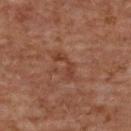Q: Is there a histopathology result?
A: total-body-photography surveillance lesion; no biopsy
Q: Lesion location?
A: the upper back
Q: What kind of image is this?
A: ~15 mm crop, total-body skin-cancer survey
Q: Patient demographics?
A: female, approximately 60 years of age